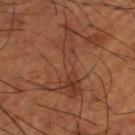workup: total-body-photography surveillance lesion; no biopsy
imaging modality: ~15 mm crop, total-body skin-cancer survey
diameter: about 9 mm
subject: male, aged around 65
illumination: cross-polarized
location: the leg